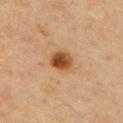follow-up: imaged on a skin check; not biopsied
automated metrics: a lesion area of about 6.5 mm², an outline eccentricity of about 0.25 (0 = round, 1 = elongated), and two-axis asymmetry of about 0.15
subject: female, aged around 45
lesion size: about 3 mm
image: 15 mm crop, total-body photography
anatomic site: the right upper arm
lighting: cross-polarized illumination